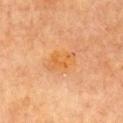Findings:
- follow-up: catalogued during a skin exam; not biopsied
- illumination: cross-polarized illumination
- diameter: about 4 mm
- location: the chest
- patient: female, approximately 60 years of age
- image: ~15 mm crop, total-body skin-cancer survey